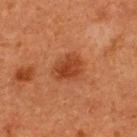| field | value |
|---|---|
| biopsy status | no biopsy performed (imaged during a skin exam) |
| body site | the back |
| lesion size | ≈3.5 mm |
| image-analysis metrics | a mean CIELAB color near L≈34 a*≈24 b*≈31, a lesion–skin lightness drop of about 8, and a normalized lesion–skin contrast near 7.5; a border-irregularity rating of about 2/10 and a peripheral color-asymmetry measure near 1; an automated nevus-likeness rating near 85 out of 100 |
| lighting | cross-polarized |
| subject | male, aged 48–52 |
| image source | ~15 mm tile from a whole-body skin photo |